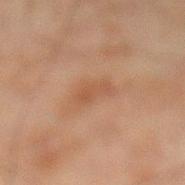Image and clinical context: This is a cross-polarized tile. The lesion is located on the left lower leg. A male patient approximately 45 years of age. About 3.5 mm across. Cropped from a total-body skin-imaging series; the visible field is about 15 mm.